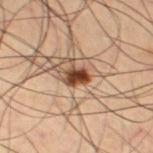Clinical impression:
No biopsy was performed on this lesion — it was imaged during a full skin examination and was not determined to be concerning.
Image and clinical context:
The lesion is on the left thigh. The total-body-photography lesion software estimated an average lesion color of about L≈43 a*≈22 b*≈32 (CIELAB). And it measured a border-irregularity rating of about 2/10, internal color variation of about 4.5 on a 0–10 scale, and peripheral color asymmetry of about 1.5. It also reported a nevus-likeness score of about 100/100. The tile uses cross-polarized illumination. A male patient in their mid- to late 60s. This image is a 15 mm lesion crop taken from a total-body photograph. Approximately 2.5 mm at its widest.